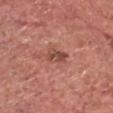Notes:
• notes: total-body-photography surveillance lesion; no biopsy
• image-analysis metrics: a lesion color around L≈48 a*≈25 b*≈27 in CIELAB, a lesion–skin lightness drop of about 10, and a normalized border contrast of about 7.5; a nevus-likeness score of about 20/100 and a lesion-detection confidence of about 100/100
• size: ~3.5 mm (longest diameter)
• body site: the head or neck
• image: 15 mm crop, total-body photography
• lighting: white-light
• patient: male, about 70 years old